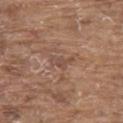notes: catalogued during a skin exam; not biopsied
image source: ~15 mm crop, total-body skin-cancer survey
patient: male, aged approximately 80
lesion size: ≈3 mm
illumination: white-light illumination
body site: the upper back